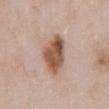<tbp_lesion>
  <biopsy_status>not biopsied; imaged during a skin examination</biopsy_status>
  <patient>
    <sex>male</sex>
    <age_approx>55</age_approx>
  </patient>
  <lesion_size>
    <long_diameter_mm_approx>5.5</long_diameter_mm_approx>
  </lesion_size>
  <image>
    <source>total-body photography crop</source>
    <field_of_view_mm>15</field_of_view_mm>
  </image>
  <automated_metrics>
    <eccentricity>0.8</eccentricity>
    <shape_asymmetry>0.2</shape_asymmetry>
    <cielab_L>54</cielab_L>
    <cielab_a>20</cielab_a>
    <cielab_b>29</cielab_b>
    <vs_skin_darker_L>15.0</vs_skin_darker_L>
    <vs_skin_contrast_norm>10.5</vs_skin_contrast_norm>
    <border_irregularity_0_10>2.0</border_irregularity_0_10>
    <color_variation_0_10>6.5</color_variation_0_10>
    <peripheral_color_asymmetry>2.5</peripheral_color_asymmetry>
  </automated_metrics>
  <site>abdomen</site>
</tbp_lesion>